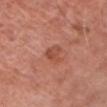diameter=~2.5 mm (longest diameter)
lighting=white-light
subject=male, roughly 80 years of age
anatomic site=the chest
image source=~15 mm tile from a whole-body skin photo